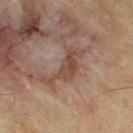Recorded during total-body skin imaging; not selected for excision or biopsy. On the right thigh. A roughly 15 mm field-of-view crop from a total-body skin photograph. The recorded lesion diameter is about 6 mm. A male patient, aged around 70.